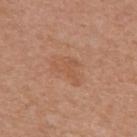<tbp_lesion>
<patient>
  <sex>female</sex>
  <age_approx>50</age_approx>
</patient>
<lesion_size>
  <long_diameter_mm_approx>4.0</long_diameter_mm_approx>
</lesion_size>
<site>left upper arm</site>
<image>
  <source>total-body photography crop</source>
  <field_of_view_mm>15</field_of_view_mm>
</image>
</tbp_lesion>